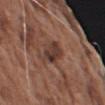The lesion was tiled from a total-body skin photograph and was not biopsied.
Captured under white-light illumination.
A close-up tile cropped from a whole-body skin photograph, about 15 mm across.
Longest diameter approximately 3.5 mm.
Automated tile analysis of the lesion measured a border-irregularity index near 2.5/10, a within-lesion color-variation index near 5.5/10, and peripheral color asymmetry of about 2.
From the left upper arm.
The subject is a male aged around 75.